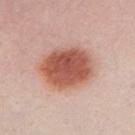  patient:
    sex: female
    age_approx: 20
  image:
    source: total-body photography crop
    field_of_view_mm: 15
  site: chest